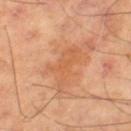This lesion was catalogued during total-body skin photography and was not selected for biopsy.
Located on the right thigh.
A 15 mm close-up tile from a total-body photography series done for melanoma screening.
The subject is a male approximately 70 years of age.
The tile uses cross-polarized illumination.
Approximately 6 mm at its widest.